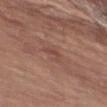{"biopsy_status": "not biopsied; imaged during a skin examination", "image": {"source": "total-body photography crop", "field_of_view_mm": 15}, "lesion_size": {"long_diameter_mm_approx": 2.5}, "automated_metrics": {"color_variation_0_10": 0.0, "peripheral_color_asymmetry": 0.0}, "patient": {"sex": "female", "age_approx": 65}, "lighting": "white-light", "site": "abdomen"}